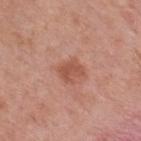| field | value |
|---|---|
| follow-up | imaged on a skin check; not biopsied |
| subject | female, aged 58–62 |
| tile lighting | white-light |
| anatomic site | the back |
| lesion diameter | ~3 mm (longest diameter) |
| image source | ~15 mm crop, total-body skin-cancer survey |